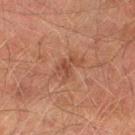{
  "image": {
    "source": "total-body photography crop",
    "field_of_view_mm": 15
  },
  "patient": {
    "sex": "male",
    "age_approx": 75
  },
  "site": "right thigh"
}